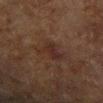Clinical impression: This lesion was catalogued during total-body skin photography and was not selected for biopsy. Image and clinical context: This is a cross-polarized tile. Longest diameter approximately 3.5 mm. Automated image analysis of the tile measured an average lesion color of about L≈24 a*≈16 b*≈20 (CIELAB), about 5 CIELAB-L* units darker than the surrounding skin, and a normalized lesion–skin contrast near 6.5. And it measured border irregularity of about 5.5 on a 0–10 scale, internal color variation of about 2.5 on a 0–10 scale, and a peripheral color-asymmetry measure near 1. A 15 mm crop from a total-body photograph taken for skin-cancer surveillance. On the right forearm. A female subject, roughly 80 years of age.